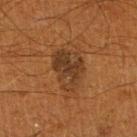This lesion was catalogued during total-body skin photography and was not selected for biopsy. A male patient aged 58 to 62. A region of skin cropped from a whole-body photographic capture, roughly 15 mm wide. Located on the leg. Captured under cross-polarized illumination. The total-body-photography lesion software estimated an area of roughly 14 mm². The software also gave roughly 7 lightness units darker than nearby skin and a lesion-to-skin contrast of about 8 (normalized; higher = more distinct). And it measured an automated nevus-likeness rating near 50 out of 100 and a detector confidence of about 100 out of 100 that the crop contains a lesion.